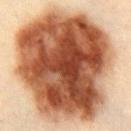Impression:
No biopsy was performed on this lesion — it was imaged during a full skin examination and was not determined to be concerning.
Image and clinical context:
From the chest. A female patient, aged 33–37. A lesion tile, about 15 mm wide, cut from a 3D total-body photograph.